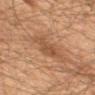This lesion was catalogued during total-body skin photography and was not selected for biopsy. Cropped from a total-body skin-imaging series; the visible field is about 15 mm. About 4.5 mm across. From the mid back. A male subject, aged 58 to 62. The tile uses cross-polarized illumination.